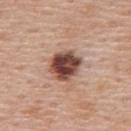Imaged during a routine full-body skin examination; the lesion was not biopsied and no histopathology is available. The tile uses white-light illumination. The total-body-photography lesion software estimated a border-irregularity index near 1.5/10, a within-lesion color-variation index near 7.5/10, and peripheral color asymmetry of about 2.5. A male patient in their 60s. The lesion is on the back. Cropped from a total-body skin-imaging series; the visible field is about 15 mm.